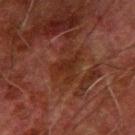This lesion was catalogued during total-body skin photography and was not selected for biopsy. A roughly 15 mm field-of-view crop from a total-body skin photograph. Longest diameter approximately 4 mm. A male patient, aged around 80. Imaged with cross-polarized lighting. On the left forearm.